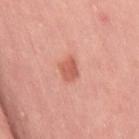Q: Was this lesion biopsied?
A: catalogued during a skin exam; not biopsied
Q: What did automated image analysis measure?
A: a footprint of about 4.5 mm² and two-axis asymmetry of about 0.3; an average lesion color of about L≈59 a*≈30 b*≈31 (CIELAB), roughly 11 lightness units darker than nearby skin, and a lesion-to-skin contrast of about 7 (normalized; higher = more distinct); a border-irregularity index near 3/10, a within-lesion color-variation index near 2/10, and peripheral color asymmetry of about 1; a classifier nevus-likeness of about 90/100 and lesion-presence confidence of about 100/100
Q: Where on the body is the lesion?
A: the right thigh
Q: Illumination type?
A: white-light
Q: Who is the patient?
A: female, aged 48 to 52
Q: How was this image acquired?
A: ~15 mm tile from a whole-body skin photo